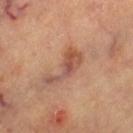<tbp_lesion>
  <biopsy_status>not biopsied; imaged during a skin examination</biopsy_status>
  <patient>
    <age_approx>60</age_approx>
  </patient>
  <image>
    <source>total-body photography crop</source>
    <field_of_view_mm>15</field_of_view_mm>
  </image>
  <site>left thigh</site>
  <lesion_size>
    <long_diameter_mm_approx>5.0</long_diameter_mm_approx>
  </lesion_size>
  <lighting>cross-polarized</lighting>
</tbp_lesion>